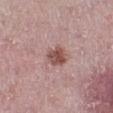<record>
  <biopsy_status>not biopsied; imaged during a skin examination</biopsy_status>
  <image>
    <source>total-body photography crop</source>
    <field_of_view_mm>15</field_of_view_mm>
  </image>
  <site>left lower leg</site>
  <patient>
    <sex>female</sex>
    <age_approx>45</age_approx>
  </patient>
</record>